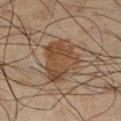Clinical impression:
No biopsy was performed on this lesion — it was imaged during a full skin examination and was not determined to be concerning.
Image and clinical context:
A 15 mm crop from a total-body photograph taken for skin-cancer surveillance. The lesion is located on the right lower leg. Imaged with cross-polarized lighting. A male subject, aged 38 to 42. The total-body-photography lesion software estimated a footprint of about 18 mm², an outline eccentricity of about 0.75 (0 = round, 1 = elongated), and a shape-asymmetry score of about 0.25 (0 = symmetric). And it measured border irregularity of about 3 on a 0–10 scale, a color-variation rating of about 4.5/10, and a peripheral color-asymmetry measure near 1.5.